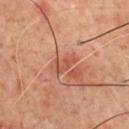workup = catalogued during a skin exam; not biopsied
image = ~15 mm tile from a whole-body skin photo
site = the chest
tile lighting = cross-polarized
size = ≈2.5 mm
subject = male, aged 68 to 72